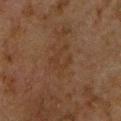| field | value |
|---|---|
| notes | no biopsy performed (imaged during a skin exam) |
| site | the chest |
| subject | male, aged 48 to 52 |
| lesion size | ≈3 mm |
| imaging modality | ~15 mm crop, total-body skin-cancer survey |
| illumination | cross-polarized illumination |
| automated metrics | a detector confidence of about 100 out of 100 that the crop contains a lesion |